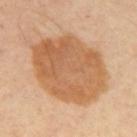Imaged during a routine full-body skin examination; the lesion was not biopsied and no histopathology is available. A close-up tile cropped from a whole-body skin photograph, about 15 mm across. The lesion is located on the upper back. A male patient, approximately 55 years of age.